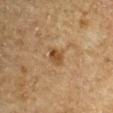subject = male, about 65 years old
size = about 2.5 mm
tile lighting = cross-polarized illumination
anatomic site = the left upper arm
image = 15 mm crop, total-body photography